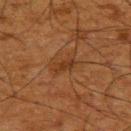biopsy_status: not biopsied; imaged during a skin examination
automated_metrics:
  cielab_L: 28
  cielab_a: 19
  cielab_b: 30
  vs_skin_darker_L: 7.0
  vs_skin_contrast_norm: 7.0
  lesion_detection_confidence_0_100: 95
image:
  source: total-body photography crop
  field_of_view_mm: 15
lesion_size:
  long_diameter_mm_approx: 2.5
site: upper back
patient:
  sex: male
  age_approx: 65